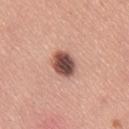Clinical summary:
The patient is a female roughly 30 years of age. An algorithmic analysis of the crop reported an area of roughly 7.5 mm², a shape eccentricity near 0.7, and a symmetry-axis asymmetry near 0.2. The software also gave a lesion color around L≈50 a*≈22 b*≈25 in CIELAB, a lesion–skin lightness drop of about 20, and a normalized border contrast of about 13. And it measured a nevus-likeness score of about 90/100 and a detector confidence of about 100 out of 100 that the crop contains a lesion. On the back. Cropped from a total-body skin-imaging series; the visible field is about 15 mm.